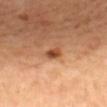This lesion was catalogued during total-body skin photography and was not selected for biopsy.
The lesion is located on the mid back.
The lesion-visualizer software estimated an eccentricity of roughly 0.75 and a shape-asymmetry score of about 0.25 (0 = symmetric). And it measured a border-irregularity rating of about 2/10 and radial color variation of about 1.
A female subject, aged 63 to 67.
This image is a 15 mm lesion crop taken from a total-body photograph.